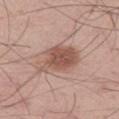Recorded during total-body skin imaging; not selected for excision or biopsy. A close-up tile cropped from a whole-body skin photograph, about 15 mm across. The patient is a male approximately 40 years of age. From the left thigh. Imaged with white-light lighting.